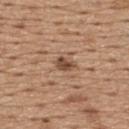biopsy_status: not biopsied; imaged during a skin examination
lighting: white-light
image:
  source: total-body photography crop
  field_of_view_mm: 15
automated_metrics:
  area_mm2_approx: 3.5
  eccentricity: 0.75
  shape_asymmetry: 0.3
  vs_skin_darker_L: 12.0
  vs_skin_contrast_norm: 9.0
  border_irregularity_0_10: 2.5
  color_variation_0_10: 3.0
  peripheral_color_asymmetry: 1.0
patient:
  sex: male
  age_approx: 65
site: back